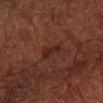Case summary:
– illumination — cross-polarized
– image — 15 mm crop, total-body photography
– anatomic site — the right forearm
– subject — male, roughly 50 years of age
– image-analysis metrics — an area of roughly 3.5 mm², an outline eccentricity of about 0.9 (0 = round, 1 = elongated), and a shape-asymmetry score of about 0.35 (0 = symmetric); a lesion color around L≈18 a*≈18 b*≈19 in CIELAB, a lesion–skin lightness drop of about 5, and a normalized lesion–skin contrast near 6; border irregularity of about 4 on a 0–10 scale, a within-lesion color-variation index near 0.5/10, and peripheral color asymmetry of about 0